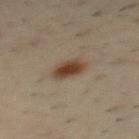Findings:
• workup: total-body-photography surveillance lesion; no biopsy
• TBP lesion metrics: a lesion area of about 6 mm² and a symmetry-axis asymmetry near 0.2; an average lesion color of about L≈40 a*≈16 b*≈29 (CIELAB), roughly 13 lightness units darker than nearby skin, and a lesion-to-skin contrast of about 11 (normalized; higher = more distinct)
• acquisition: total-body-photography crop, ~15 mm field of view
• anatomic site: the upper back
• illumination: cross-polarized illumination
• subject: male, roughly 40 years of age
• size: ≈3.5 mm